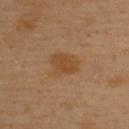The lesion is on the upper back.
A 15 mm close-up extracted from a 3D total-body photography capture.
The subject is a male aged 38 to 42.